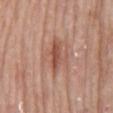Assessment:
Part of a total-body skin-imaging series; this lesion was reviewed on a skin check and was not flagged for biopsy.
Acquisition and patient details:
Located on the mid back. Cropped from a total-body skin-imaging series; the visible field is about 15 mm. Automated image analysis of the tile measured a lesion area of about 7.5 mm² and an eccentricity of roughly 0.8. The software also gave an average lesion color of about L≈52 a*≈23 b*≈29 (CIELAB). It also reported internal color variation of about 4.5 on a 0–10 scale and peripheral color asymmetry of about 1.5. A male patient in their mid- to late 70s. Approximately 4 mm at its widest.